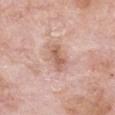follow-up: total-body-photography surveillance lesion; no biopsy
imaging modality: ~15 mm tile from a whole-body skin photo
diameter: ~4 mm (longest diameter)
image-analysis metrics: a footprint of about 5.5 mm², a shape eccentricity near 0.9, and two-axis asymmetry of about 0.25; border irregularity of about 3 on a 0–10 scale, a color-variation rating of about 2.5/10, and a peripheral color-asymmetry measure near 1
anatomic site: the chest
tile lighting: white-light illumination
patient: female, in their mid- to late 70s